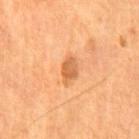{
  "biopsy_status": "not biopsied; imaged during a skin examination",
  "patient": {
    "sex": "male",
    "age_approx": 55
  },
  "image": {
    "source": "total-body photography crop",
    "field_of_view_mm": 15
  },
  "site": "chest"
}